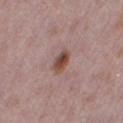follow-up: no biopsy performed (imaged during a skin exam) | tile lighting: white-light | subject: female, aged around 30 | lesion size: about 3 mm | acquisition: ~15 mm tile from a whole-body skin photo | site: the leg.